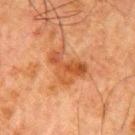| feature | finding |
|---|---|
| biopsy status | imaged on a skin check; not biopsied |
| patient | male, roughly 80 years of age |
| site | the left upper arm |
| acquisition | total-body-photography crop, ~15 mm field of view |